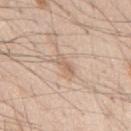Clinical impression: Imaged during a routine full-body skin examination; the lesion was not biopsied and no histopathology is available. Acquisition and patient details: On the mid back. Cropped from a total-body skin-imaging series; the visible field is about 15 mm. Imaged with white-light lighting. The lesion-visualizer software estimated a footprint of about 3 mm² and a shape eccentricity near 0.9. A male patient about 45 years old. About 3 mm across.